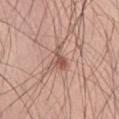The lesion was photographed on a routine skin check and not biopsied; there is no pathology result.
A region of skin cropped from a whole-body photographic capture, roughly 15 mm wide.
An algorithmic analysis of the crop reported internal color variation of about 3.5 on a 0–10 scale.
Measured at roughly 3 mm in maximum diameter.
Located on the abdomen.
A male subject, aged 58–62.